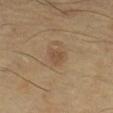location: the right lower leg | subject: female, approximately 70 years of age | size: ~2 mm (longest diameter) | acquisition: ~15 mm tile from a whole-body skin photo | automated metrics: a border-irregularity rating of about 3/10 and a within-lesion color-variation index near 2/10.